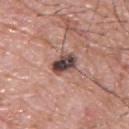notes — imaged on a skin check; not biopsied
patient — male, approximately 55 years of age
TBP lesion metrics — an outline eccentricity of about 0.65 (0 = round, 1 = elongated) and a symmetry-axis asymmetry near 0.2; a mean CIELAB color near L≈44 a*≈18 b*≈20, roughly 18 lightness units darker than nearby skin, and a normalized lesion–skin contrast near 13; a border-irregularity rating of about 2/10, a within-lesion color-variation index near 8/10, and a peripheral color-asymmetry measure near 2.5; a detector confidence of about 100 out of 100 that the crop contains a lesion
lighting — white-light
acquisition — ~15 mm tile from a whole-body skin photo